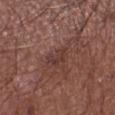<lesion>
<biopsy_status>not biopsied; imaged during a skin examination</biopsy_status>
<patient>
  <sex>male</sex>
  <age_approx>65</age_approx>
</patient>
<image>
  <source>total-body photography crop</source>
  <field_of_view_mm>15</field_of_view_mm>
</image>
<automated_metrics>
  <eccentricity>0.85</eccentricity>
  <shape_asymmetry>0.45</shape_asymmetry>
  <cielab_L>36</cielab_L>
  <cielab_a>20</cielab_a>
  <cielab_b>21</cielab_b>
  <vs_skin_darker_L>7.0</vs_skin_darker_L>
  <vs_skin_contrast_norm>6.5</vs_skin_contrast_norm>
  <border_irregularity_0_10>5.5</border_irregularity_0_10>
  <color_variation_0_10>0.0</color_variation_0_10>
  <nevus_likeness_0_100>0</nevus_likeness_0_100>
  <lesion_detection_confidence_0_100>80</lesion_detection_confidence_0_100>
</automated_metrics>
<lighting>white-light</lighting>
<site>left lower leg</site>
</lesion>